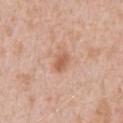Captured during whole-body skin photography for melanoma surveillance; the lesion was not biopsied. The tile uses white-light illumination. Located on the back. Cropped from a total-body skin-imaging series; the visible field is about 15 mm. Automated image analysis of the tile measured a shape eccentricity near 0.75 and a symmetry-axis asymmetry near 0.2. It also reported a border-irregularity index near 1.5/10, a within-lesion color-variation index near 2/10, and a peripheral color-asymmetry measure near 0.5. And it measured an automated nevus-likeness rating near 20 out of 100 and a detector confidence of about 100 out of 100 that the crop contains a lesion. A male subject, aged 58 to 62. The recorded lesion diameter is about 2.5 mm.